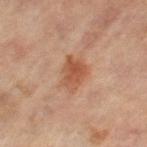follow-up — catalogued during a skin exam; not biopsied | body site — the right leg | image-analysis metrics — a footprint of about 8 mm², an outline eccentricity of about 0.7 (0 = round, 1 = elongated), and two-axis asymmetry of about 0.2; border irregularity of about 2.5 on a 0–10 scale, a color-variation rating of about 3.5/10, and radial color variation of about 1; a classifier nevus-likeness of about 75/100 and a detector confidence of about 100 out of 100 that the crop contains a lesion | image source — 15 mm crop, total-body photography | diameter — ≈4 mm | subject — female, in their mid-60s | illumination — cross-polarized.